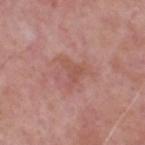Notes:
• follow-up: imaged on a skin check; not biopsied
• acquisition: total-body-photography crop, ~15 mm field of view
• location: the head or neck
• tile lighting: white-light illumination
• automated metrics: a mean CIELAB color near L≈53 a*≈24 b*≈26, roughly 6 lightness units darker than nearby skin, and a lesion-to-skin contrast of about 5 (normalized; higher = more distinct); a nevus-likeness score of about 0/100 and a lesion-detection confidence of about 100/100
• subject: male, aged around 70